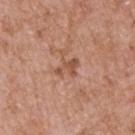A 15 mm close-up extracted from a 3D total-body photography capture.
The lesion's longest dimension is about 3 mm.
Imaged with white-light lighting.
The total-body-photography lesion software estimated a lesion area of about 4.5 mm², a shape eccentricity near 0.7, and two-axis asymmetry of about 0.25. The software also gave about 9 CIELAB-L* units darker than the surrounding skin. The analysis additionally found an automated nevus-likeness rating near 0 out of 100 and lesion-presence confidence of about 100/100.
Located on the upper back.
The subject is a male in their 60s.